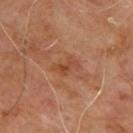<tbp_lesion>
  <biopsy_status>not biopsied; imaged during a skin examination</biopsy_status>
  <site>upper back</site>
  <image>
    <source>total-body photography crop</source>
    <field_of_view_mm>15</field_of_view_mm>
  </image>
  <patient>
    <sex>male</sex>
    <age_approx>65</age_approx>
  </patient>
</tbp_lesion>